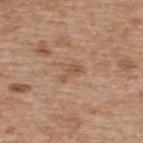The lesion was photographed on a routine skin check and not biopsied; there is no pathology result. A male subject, approximately 65 years of age. The tile uses white-light illumination. Cropped from a total-body skin-imaging series; the visible field is about 15 mm. The lesion is located on the upper back. The total-body-photography lesion software estimated a lesion area of about 3 mm² and an eccentricity of roughly 0.9. The software also gave a mean CIELAB color near L≈53 a*≈20 b*≈32 and a normalized border contrast of about 6. The analysis additionally found a border-irregularity index near 5/10, a color-variation rating of about 1/10, and a peripheral color-asymmetry measure near 0. It also reported a classifier nevus-likeness of about 0/100. Longest diameter approximately 3 mm.